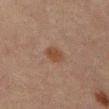Clinical impression: The lesion was tiled from a total-body skin photograph and was not biopsied. Acquisition and patient details: A lesion tile, about 15 mm wide, cut from a 3D total-body photograph. From the mid back. A male subject about 65 years old.